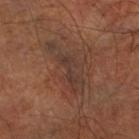| key | value |
|---|---|
| workup | total-body-photography surveillance lesion; no biopsy |
| subject | aged 63–67 |
| imaging modality | total-body-photography crop, ~15 mm field of view |
| location | the leg |